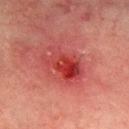| feature | finding |
|---|---|
| workup | no biopsy performed (imaged during a skin exam) |
| site | the right lower leg |
| diameter | ≈5 mm |
| lighting | cross-polarized |
| imaging modality | ~15 mm crop, total-body skin-cancer survey |
| patient | male, aged around 75 |